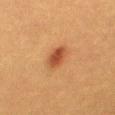A female subject, aged 38–42. Imaged with cross-polarized lighting. Cropped from a total-body skin-imaging series; the visible field is about 15 mm. The lesion is on the right thigh. Automated tile analysis of the lesion measured border irregularity of about 1.5 on a 0–10 scale and peripheral color asymmetry of about 1.